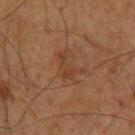  biopsy_status: not biopsied; imaged during a skin examination
  patient:
    sex: male
    age_approx: 60
  lesion_size:
    long_diameter_mm_approx: 3.5
  site: upper back
  image:
    source: total-body photography crop
    field_of_view_mm: 15